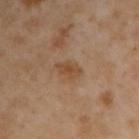Findings:
* follow-up · catalogued during a skin exam; not biopsied
* lesion size · ≈3 mm
* acquisition · 15 mm crop, total-body photography
* automated lesion analysis · a border-irregularity rating of about 3/10 and a peripheral color-asymmetry measure near 0.5; a detector confidence of about 100 out of 100 that the crop contains a lesion
* site · the right upper arm
* subject · male, aged approximately 55